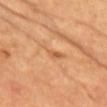Q: Was a biopsy performed?
A: total-body-photography surveillance lesion; no biopsy
Q: Patient demographics?
A: female, roughly 40 years of age
Q: Lesion size?
A: ~4 mm (longest diameter)
Q: What did automated image analysis measure?
A: a footprint of about 4.5 mm², an eccentricity of roughly 0.95, and a shape-asymmetry score of about 0.55 (0 = symmetric); border irregularity of about 6 on a 0–10 scale, a within-lesion color-variation index near 2.5/10, and peripheral color asymmetry of about 1
Q: What is the imaging modality?
A: ~15 mm crop, total-body skin-cancer survey
Q: What lighting was used for the tile?
A: cross-polarized
Q: What is the anatomic site?
A: the chest